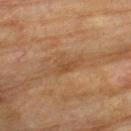Clinical impression:
Imaged during a routine full-body skin examination; the lesion was not biopsied and no histopathology is available.
Image and clinical context:
An algorithmic analysis of the crop reported a footprint of about 5.5 mm², an eccentricity of roughly 0.9, and a shape-asymmetry score of about 0.35 (0 = symmetric). The software also gave roughly 6 lightness units darker than nearby skin. The analysis additionally found border irregularity of about 4.5 on a 0–10 scale and radial color variation of about 0.5. The analysis additionally found an automated nevus-likeness rating near 0 out of 100 and lesion-presence confidence of about 75/100. This is a cross-polarized tile. From the upper back. Measured at roughly 4.5 mm in maximum diameter. A male subject, about 75 years old. Cropped from a whole-body photographic skin survey; the tile spans about 15 mm.